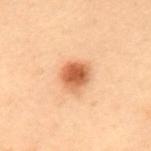Q: Was a biopsy performed?
A: imaged on a skin check; not biopsied